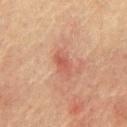Clinical impression:
This lesion was catalogued during total-body skin photography and was not selected for biopsy.
Acquisition and patient details:
A 15 mm close-up extracted from a 3D total-body photography capture. A male subject approximately 65 years of age. From the chest. About 3 mm across.